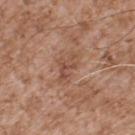The lesion was photographed on a routine skin check and not biopsied; there is no pathology result. The lesion is on the upper back. A 15 mm close-up tile from a total-body photography series done for melanoma screening. The subject is a male in their mid-50s. This is a white-light tile. The recorded lesion diameter is about 3.5 mm. The lesion-visualizer software estimated a footprint of about 5.5 mm², an outline eccentricity of about 0.8 (0 = round, 1 = elongated), and two-axis asymmetry of about 0.5.